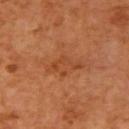notes — imaged on a skin check; not biopsied | imaging modality — total-body-photography crop, ~15 mm field of view | location — the arm | lighting — cross-polarized | patient — aged approximately 65.